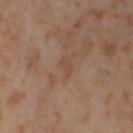Findings:
– follow-up: no biopsy performed (imaged during a skin exam)
– subject: female, in their mid-50s
– acquisition: total-body-photography crop, ~15 mm field of view
– illumination: cross-polarized
– lesion diameter: about 2.5 mm
– site: the leg